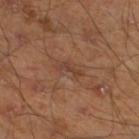Q: Was a biopsy performed?
A: no biopsy performed (imaged during a skin exam)
Q: What are the patient's age and sex?
A: male, in their mid-40s
Q: How large is the lesion?
A: ~2.5 mm (longest diameter)
Q: Automated lesion metrics?
A: a lesion–skin lightness drop of about 6 and a normalized lesion–skin contrast near 5.5
Q: What is the imaging modality?
A: ~15 mm tile from a whole-body skin photo
Q: Lesion location?
A: the left lower leg
Q: What lighting was used for the tile?
A: cross-polarized illumination